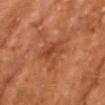Assessment: This lesion was catalogued during total-body skin photography and was not selected for biopsy. Context: An algorithmic analysis of the crop reported a shape eccentricity near 0.75 and a symmetry-axis asymmetry near 0.4. Cropped from a whole-body photographic skin survey; the tile spans about 15 mm. Captured under cross-polarized illumination. On the chest. The subject is a male in their 70s.